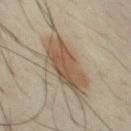Q: Is there a histopathology result?
A: imaged on a skin check; not biopsied
Q: What are the patient's age and sex?
A: male, in their 50s
Q: What is the imaging modality?
A: 15 mm crop, total-body photography
Q: Lesion size?
A: about 7 mm
Q: Automated lesion metrics?
A: an area of roughly 18 mm² and a shape-asymmetry score of about 0.2 (0 = symmetric); an average lesion color of about L≈47 a*≈12 b*≈27 (CIELAB), roughly 10 lightness units darker than nearby skin, and a normalized border contrast of about 8.5; an automated nevus-likeness rating near 95 out of 100 and lesion-presence confidence of about 80/100
Q: What is the anatomic site?
A: the chest